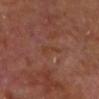The lesion was tiled from a total-body skin photograph and was not biopsied. A male subject aged 63 to 67. The lesion is on the head or neck. A lesion tile, about 15 mm wide, cut from a 3D total-body photograph.